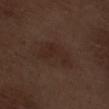<tbp_lesion>
  <patient>
    <sex>male</sex>
    <age_approx>70</age_approx>
  </patient>
  <image>
    <source>total-body photography crop</source>
    <field_of_view_mm>15</field_of_view_mm>
  </image>
  <lighting>white-light</lighting>
  <lesion_size>
    <long_diameter_mm_approx>4.5</long_diameter_mm_approx>
  </lesion_size>
  <automated_metrics>
    <cielab_L>25</cielab_L>
    <cielab_a>16</cielab_a>
    <cielab_b>22</cielab_b>
    <vs_skin_darker_L>5.0</vs_skin_darker_L>
    <vs_skin_contrast_norm>5.5</vs_skin_contrast_norm>
    <lesion_detection_confidence_0_100>100</lesion_detection_confidence_0_100>
  </automated_metrics>
  <site>right thigh</site>
</tbp_lesion>